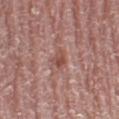Captured during whole-body skin photography for melanoma surveillance; the lesion was not biopsied. Captured under white-light illumination. Automated tile analysis of the lesion measured a lesion area of about 3 mm², an eccentricity of roughly 0.75, and a shape-asymmetry score of about 0.4 (0 = symmetric). And it measured lesion-presence confidence of about 100/100. Measured at roughly 2.5 mm in maximum diameter. The subject is a female aged 63 to 67. The lesion is located on the left thigh. Cropped from a total-body skin-imaging series; the visible field is about 15 mm.